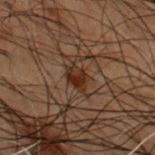Imaged during a routine full-body skin examination; the lesion was not biopsied and no histopathology is available. A close-up tile cropped from a whole-body skin photograph, about 15 mm across. This is a cross-polarized tile. Located on the back. The subject is a male aged 48 to 52.